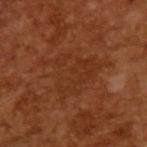Impression: The lesion was photographed on a routine skin check and not biopsied; there is no pathology result. Clinical summary: The lesion-visualizer software estimated a footprint of about 20 mm², an outline eccentricity of about 0.6 (0 = round, 1 = elongated), and two-axis asymmetry of about 0.5. The software also gave a mean CIELAB color near L≈32 a*≈24 b*≈32, a lesion–skin lightness drop of about 5, and a lesion-to-skin contrast of about 4.5 (normalized; higher = more distinct). It also reported a classifier nevus-likeness of about 0/100 and lesion-presence confidence of about 75/100. Captured under cross-polarized illumination. Cropped from a total-body skin-imaging series; the visible field is about 15 mm. A male subject, about 65 years old.